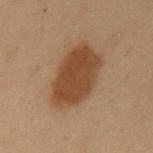Impression:
Part of a total-body skin-imaging series; this lesion was reviewed on a skin check and was not flagged for biopsy.
Background:
Captured under cross-polarized illumination. From the mid back. A male patient, about 55 years old. A lesion tile, about 15 mm wide, cut from a 3D total-body photograph. Measured at roughly 7 mm in maximum diameter. Automated image analysis of the tile measured an area of roughly 22 mm², a shape eccentricity near 0.85, and a symmetry-axis asymmetry near 0.15. The software also gave an average lesion color of about L≈36 a*≈17 b*≈28 (CIELAB). The analysis additionally found a border-irregularity rating of about 2/10 and a color-variation rating of about 2.5/10. And it measured a classifier nevus-likeness of about 100/100.